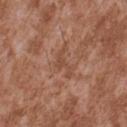Assessment:
The lesion was photographed on a routine skin check and not biopsied; there is no pathology result.
Context:
A roughly 15 mm field-of-view crop from a total-body skin photograph. An algorithmic analysis of the crop reported a lesion area of about 5 mm² and a shape-asymmetry score of about 0.75 (0 = symmetric). And it measured an average lesion color of about L≈48 a*≈22 b*≈30 (CIELAB). It also reported a color-variation rating of about 1/10 and peripheral color asymmetry of about 0.5. A male subject roughly 45 years of age. About 4 mm across. The tile uses white-light illumination. The lesion is located on the upper back.